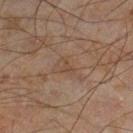Cropped from a whole-body photographic skin survey; the tile spans about 15 mm.
Located on the right lower leg.
Measured at roughly 2.5 mm in maximum diameter.
The subject is a male aged 43 to 47.